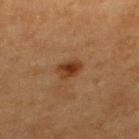notes: catalogued during a skin exam; not biopsied
body site: the upper back
image source: total-body-photography crop, ~15 mm field of view
illumination: cross-polarized illumination
TBP lesion metrics: an area of roughly 4.5 mm² and a shape eccentricity near 0.7; an automated nevus-likeness rating near 95 out of 100 and lesion-presence confidence of about 100/100
subject: female, about 40 years old